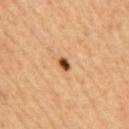notes — no biopsy performed (imaged during a skin exam)
subject — male, approximately 60 years of age
site — the mid back
image — 15 mm crop, total-body photography
lighting — cross-polarized illumination
diameter — ≈2 mm
automated metrics — a lesion–skin lightness drop of about 20 and a lesion-to-skin contrast of about 13.5 (normalized; higher = more distinct); an automated nevus-likeness rating near 100 out of 100 and a lesion-detection confidence of about 100/100